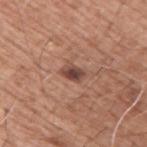* biopsy status: no biopsy performed (imaged during a skin exam)
* size: ~3 mm (longest diameter)
* patient: male, roughly 60 years of age
* imaging modality: ~15 mm tile from a whole-body skin photo
* tile lighting: white-light illumination
* location: the arm